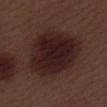No biopsy was performed on this lesion — it was imaged during a full skin examination and was not determined to be concerning. This image is a 15 mm lesion crop taken from a total-body photograph. Measured at roughly 8.5 mm in maximum diameter. A male patient approximately 70 years of age. On the right lower leg. The tile uses white-light illumination.